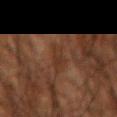Notes:
– workup — total-body-photography surveillance lesion; no biopsy
– acquisition — ~15 mm tile from a whole-body skin photo
– illumination — cross-polarized illumination
– diameter — ≈3.5 mm
– automated lesion analysis — a shape eccentricity near 0.8 and two-axis asymmetry of about 0.3; border irregularity of about 4 on a 0–10 scale, a within-lesion color-variation index near 2.5/10, and a peripheral color-asymmetry measure near 1
– patient — male, roughly 65 years of age
– location — the left forearm